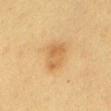<lesion>
  <biopsy_status>not biopsied; imaged during a skin examination</biopsy_status>
  <patient>
    <sex>female</sex>
    <age_approx>30</age_approx>
  </patient>
  <image>
    <source>total-body photography crop</source>
    <field_of_view_mm>15</field_of_view_mm>
  </image>
  <site>mid back</site>
</lesion>